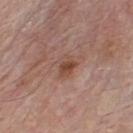Q: Was this lesion biopsied?
A: no biopsy performed (imaged during a skin exam)
Q: What did automated image analysis measure?
A: a mean CIELAB color near L≈47 a*≈20 b*≈27, a lesion–skin lightness drop of about 9, and a normalized border contrast of about 7.5
Q: Illumination type?
A: white-light
Q: What is the imaging modality?
A: ~15 mm crop, total-body skin-cancer survey
Q: Patient demographics?
A: male, aged 78 to 82
Q: What is the anatomic site?
A: the chest